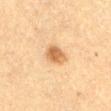location: the front of the torso
image source: ~15 mm tile from a whole-body skin photo
patient: female, in their mid- to late 50s
illumination: cross-polarized
size: ~3 mm (longest diameter)